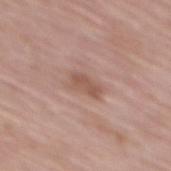Part of a total-body skin-imaging series; this lesion was reviewed on a skin check and was not flagged for biopsy.
The lesion-visualizer software estimated an automated nevus-likeness rating near 0 out of 100 and a lesion-detection confidence of about 100/100.
Cropped from a whole-body photographic skin survey; the tile spans about 15 mm.
The lesion is located on the mid back.
A female patient, aged around 60.
Captured under white-light illumination.